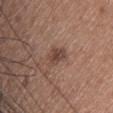Recorded during total-body skin imaging; not selected for excision or biopsy.
From the head or neck.
A female patient approximately 60 years of age.
Automated image analysis of the tile measured an area of roughly 4.5 mm², a shape eccentricity near 0.75, and a symmetry-axis asymmetry near 0.25. The software also gave border irregularity of about 2.5 on a 0–10 scale, a within-lesion color-variation index near 4/10, and a peripheral color-asymmetry measure near 1.5. The software also gave a nevus-likeness score of about 45/100 and a detector confidence of about 100 out of 100 that the crop contains a lesion.
A lesion tile, about 15 mm wide, cut from a 3D total-body photograph.